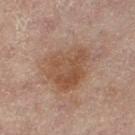No biopsy was performed on this lesion — it was imaged during a full skin examination and was not determined to be concerning.
A close-up tile cropped from a whole-body skin photograph, about 15 mm across.
The total-body-photography lesion software estimated a lesion area of about 22 mm² and an outline eccentricity of about 0.6 (0 = round, 1 = elongated). It also reported an average lesion color of about L≈46 a*≈17 b*≈27 (CIELAB), a lesion–skin lightness drop of about 8, and a normalized lesion–skin contrast near 7.
A female patient, aged 78–82.
On the right leg.
About 6.5 mm across.